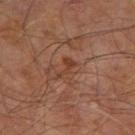{
  "biopsy_status": "not biopsied; imaged during a skin examination",
  "patient": {
    "sex": "male",
    "age_approx": 60
  },
  "site": "right leg",
  "image": {
    "source": "total-body photography crop",
    "field_of_view_mm": 15
  }
}